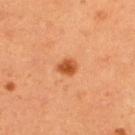Clinical impression:
This lesion was catalogued during total-body skin photography and was not selected for biopsy.
Acquisition and patient details:
Automated tile analysis of the lesion measured a mean CIELAB color near L≈53 a*≈30 b*≈43 and a normalized lesion–skin contrast near 9. A male subject aged 33–37. Cropped from a total-body skin-imaging series; the visible field is about 15 mm. From the upper back.